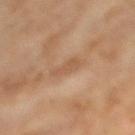This lesion was catalogued during total-body skin photography and was not selected for biopsy. Cropped from a whole-body photographic skin survey; the tile spans about 15 mm. Measured at roughly 3.5 mm in maximum diameter. A female subject, aged 68 to 72. The lesion is located on the right lower leg. Captured under cross-polarized illumination. An algorithmic analysis of the crop reported an average lesion color of about L≈52 a*≈19 b*≈32 (CIELAB) and a normalized border contrast of about 5.5.